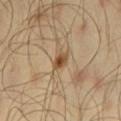Captured during whole-body skin photography for melanoma surveillance; the lesion was not biopsied. Cropped from a whole-body photographic skin survey; the tile spans about 15 mm. About 2.5 mm across. The lesion is located on the left thigh. The patient is a male aged approximately 45.